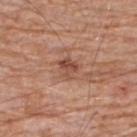follow-up: total-body-photography surveillance lesion; no biopsy
location: the upper back
automated metrics: an outline eccentricity of about 0.3 (0 = round, 1 = elongated) and a symmetry-axis asymmetry near 0.2; an average lesion color of about L≈51 a*≈22 b*≈29 (CIELAB), a lesion–skin lightness drop of about 9, and a normalized lesion–skin contrast near 6.5; an automated nevus-likeness rating near 0 out of 100
lesion diameter: about 3 mm
patient: male, aged around 80
tile lighting: white-light
image: ~15 mm crop, total-body skin-cancer survey